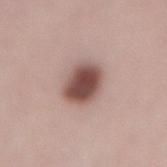Recorded during total-body skin imaging; not selected for excision or biopsy. The patient is a male approximately 40 years of age. Approximately 5 mm at its widest. The lesion-visualizer software estimated a footprint of about 11 mm², an eccentricity of roughly 0.8, and a symmetry-axis asymmetry near 0.15. The software also gave an average lesion color of about L≈49 a*≈20 b*≈22 (CIELAB), about 18 CIELAB-L* units darker than the surrounding skin, and a normalized border contrast of about 12. It also reported an automated nevus-likeness rating near 95 out of 100 and a detector confidence of about 100 out of 100 that the crop contains a lesion. Cropped from a whole-body photographic skin survey; the tile spans about 15 mm. Captured under white-light illumination. Located on the lower back.